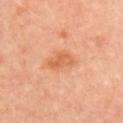Context: Located on the back. Approximately 3.5 mm at its widest. A female subject, aged 48 to 52. The lesion-visualizer software estimated a detector confidence of about 100 out of 100 that the crop contains a lesion. A roughly 15 mm field-of-view crop from a total-body skin photograph.